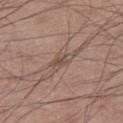<case>
  <biopsy_status>not biopsied; imaged during a skin examination</biopsy_status>
  <automated_metrics>
    <area_mm2_approx>4.0</area_mm2_approx>
    <eccentricity>0.85</eccentricity>
    <shape_asymmetry>0.25</shape_asymmetry>
    <vs_skin_darker_L>7.0</vs_skin_darker_L>
    <vs_skin_contrast_norm>5.5</vs_skin_contrast_norm>
    <border_irregularity_0_10>3.0</border_irregularity_0_10>
    <color_variation_0_10>4.0</color_variation_0_10>
    <peripheral_color_asymmetry>1.0</peripheral_color_asymmetry>
    <nevus_likeness_0_100>0</nevus_likeness_0_100>
  </automated_metrics>
  <site>right thigh</site>
  <patient>
    <sex>male</sex>
    <age_approx>55</age_approx>
  </patient>
  <lesion_size>
    <long_diameter_mm_approx>3.0</long_diameter_mm_approx>
  </lesion_size>
  <image>
    <source>total-body photography crop</source>
    <field_of_view_mm>15</field_of_view_mm>
  </image>
</case>